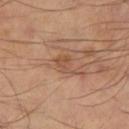Captured during whole-body skin photography for melanoma surveillance; the lesion was not biopsied. A 15 mm close-up tile from a total-body photography series done for melanoma screening. The lesion-visualizer software estimated a lesion color around L≈52 a*≈22 b*≈32 in CIELAB, a lesion–skin lightness drop of about 7, and a normalized border contrast of about 5.5. The software also gave border irregularity of about 4.5 on a 0–10 scale, internal color variation of about 2.5 on a 0–10 scale, and radial color variation of about 1. The analysis additionally found a nevus-likeness score of about 0/100 and a lesion-detection confidence of about 100/100. Approximately 2.5 mm at its widest. Located on the leg. Captured under cross-polarized illumination.